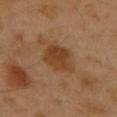Background:
This is a cross-polarized tile. The lesion is on the front of the torso. The patient is a male approximately 50 years of age. This image is a 15 mm lesion crop taken from a total-body photograph.